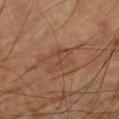Q: Was this lesion biopsied?
A: catalogued during a skin exam; not biopsied
Q: How was the tile lit?
A: cross-polarized
Q: Where on the body is the lesion?
A: the right upper arm
Q: Lesion size?
A: about 4.5 mm
Q: What kind of image is this?
A: ~15 mm crop, total-body skin-cancer survey
Q: What are the patient's age and sex?
A: male, aged approximately 60
Q: Automated lesion metrics?
A: a lesion area of about 4.5 mm², an outline eccentricity of about 0.95 (0 = round, 1 = elongated), and two-axis asymmetry of about 0.6; an average lesion color of about L≈43 a*≈20 b*≈28 (CIELAB), roughly 6 lightness units darker than nearby skin, and a lesion-to-skin contrast of about 5 (normalized; higher = more distinct); an automated nevus-likeness rating near 0 out of 100 and a detector confidence of about 100 out of 100 that the crop contains a lesion